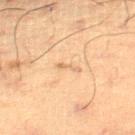Part of a total-body skin-imaging series; this lesion was reviewed on a skin check and was not flagged for biopsy.
From the right thigh.
A 15 mm close-up tile from a total-body photography series done for melanoma screening.
The patient is a male roughly 60 years of age.